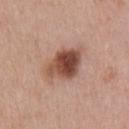Acquisition and patient details:
The total-body-photography lesion software estimated an area of roughly 14 mm², an outline eccentricity of about 0.75 (0 = round, 1 = elongated), and two-axis asymmetry of about 0.2. The software also gave a mean CIELAB color near L≈49 a*≈22 b*≈27, a lesion–skin lightness drop of about 15, and a normalized border contrast of about 10.5. A male subject, aged 48–52. The tile uses white-light illumination. On the upper back. Cropped from a total-body skin-imaging series; the visible field is about 15 mm. The lesion's longest dimension is about 5.5 mm.